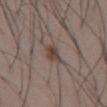{
  "biopsy_status": "not biopsied; imaged during a skin examination",
  "lighting": "white-light",
  "site": "abdomen",
  "automated_metrics": {
    "color_variation_0_10": 3.5,
    "peripheral_color_asymmetry": 1.0,
    "nevus_likeness_0_100": 95,
    "lesion_detection_confidence_0_100": 100
  },
  "image": {
    "source": "total-body photography crop",
    "field_of_view_mm": 15
  },
  "lesion_size": {
    "long_diameter_mm_approx": 3.0
  },
  "patient": {
    "sex": "male",
    "age_approx": 75
  }
}